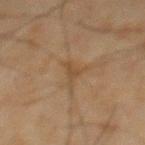Clinical impression: This lesion was catalogued during total-body skin photography and was not selected for biopsy. Acquisition and patient details: A lesion tile, about 15 mm wide, cut from a 3D total-body photograph. The lesion is on the abdomen. A male subject roughly 65 years of age.